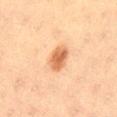{
  "biopsy_status": "not biopsied; imaged during a skin examination",
  "image": {
    "source": "total-body photography crop",
    "field_of_view_mm": 15
  },
  "automated_metrics": {
    "area_mm2_approx": 6.5,
    "eccentricity": 0.85,
    "shape_asymmetry": 0.2,
    "cielab_L": 56,
    "cielab_a": 23,
    "cielab_b": 35,
    "vs_skin_darker_L": 12.0,
    "vs_skin_contrast_norm": 8.5,
    "color_variation_0_10": 3.5,
    "peripheral_color_asymmetry": 1.0,
    "nevus_likeness_0_100": 100,
    "lesion_detection_confidence_0_100": 100
  },
  "site": "left thigh",
  "patient": {
    "sex": "female",
    "age_approx": 40
  }
}